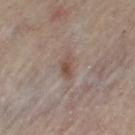Case summary:
• body site: the left thigh
• subject: female, aged 58 to 62
• acquisition: total-body-photography crop, ~15 mm field of view